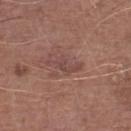The lesion was photographed on a routine skin check and not biopsied; there is no pathology result.
A region of skin cropped from a whole-body photographic capture, roughly 15 mm wide.
The lesion is on the left lower leg.
The patient is a male aged approximately 75.